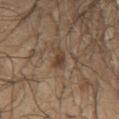This lesion was catalogued during total-body skin photography and was not selected for biopsy.
A male subject, about 50 years old.
A roughly 15 mm field-of-view crop from a total-body skin photograph.
Longest diameter approximately 3 mm.
Captured under cross-polarized illumination.
From the chest.